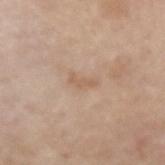Notes:
- notes · no biopsy performed (imaged during a skin exam)
- image source · ~15 mm tile from a whole-body skin photo
- location · the left forearm
- subject · female, in their mid-60s